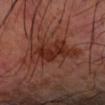Image and clinical context:
The lesion is on the left forearm. A 15 mm close-up extracted from a 3D total-body photography capture. This is a cross-polarized tile. A male subject, aged around 70. Longest diameter approximately 7 mm. An algorithmic analysis of the crop reported a lesion area of about 18 mm², an outline eccentricity of about 0.85 (0 = round, 1 = elongated), and a shape-asymmetry score of about 0.35 (0 = symmetric). The analysis additionally found a border-irregularity rating of about 5/10. The analysis additionally found a classifier nevus-likeness of about 45/100.